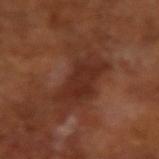biopsy_status: not biopsied; imaged during a skin examination
lighting: cross-polarized
image:
  source: total-body photography crop
  field_of_view_mm: 15
patient:
  sex: male
  age_approx: 65